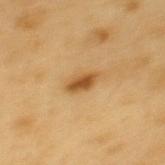Assessment:
Imaged during a routine full-body skin examination; the lesion was not biopsied and no histopathology is available.
Background:
A 15 mm close-up tile from a total-body photography series done for melanoma screening. A male subject aged 58 to 62. The lesion is located on the back.